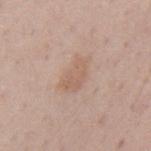Assessment: This lesion was catalogued during total-body skin photography and was not selected for biopsy. Acquisition and patient details: This image is a 15 mm lesion crop taken from a total-body photograph. An algorithmic analysis of the crop reported a mean CIELAB color near L≈61 a*≈17 b*≈28, a lesion–skin lightness drop of about 7, and a normalized border contrast of about 5. Located on the mid back. A male patient, roughly 70 years of age.